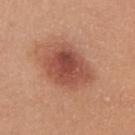Q: Was this lesion biopsied?
A: no biopsy performed (imaged during a skin exam)
Q: What lighting was used for the tile?
A: white-light illumination
Q: How was this image acquired?
A: ~15 mm crop, total-body skin-cancer survey
Q: Automated lesion metrics?
A: a color-variation rating of about 5.5/10 and peripheral color asymmetry of about 1.5
Q: Who is the patient?
A: female, roughly 45 years of age
Q: Where on the body is the lesion?
A: the chest
Q: Lesion size?
A: ≈5.5 mm